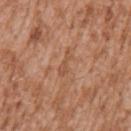Imaged during a routine full-body skin examination; the lesion was not biopsied and no histopathology is available. Located on the arm. The tile uses white-light illumination. A male patient aged approximately 45. Automated image analysis of the tile measured a mean CIELAB color near L≈52 a*≈22 b*≈33 and a normalized border contrast of about 5. And it measured a color-variation rating of about 0/10 and peripheral color asymmetry of about 0. And it measured a lesion-detection confidence of about 90/100. A 15 mm crop from a total-body photograph taken for skin-cancer surveillance.